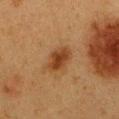Clinical impression:
The lesion was tiled from a total-body skin photograph and was not biopsied.
Context:
Captured under cross-polarized illumination. Located on the front of the torso. A female patient in their 40s. Approximately 4 mm at its widest. A 15 mm crop from a total-body photograph taken for skin-cancer surveillance.